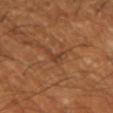Acquisition and patient details: Longest diameter approximately 3 mm. A close-up tile cropped from a whole-body skin photograph, about 15 mm across. A male patient, in their mid- to late 60s. From the right upper arm. Captured under cross-polarized illumination.